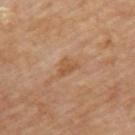Recorded during total-body skin imaging; not selected for excision or biopsy. Automated tile analysis of the lesion measured an area of roughly 3.5 mm², an outline eccentricity of about 0.8 (0 = round, 1 = elongated), and a symmetry-axis asymmetry near 0.45. And it measured a mean CIELAB color near L≈54 a*≈21 b*≈37 and roughly 8 lightness units darker than nearby skin. It also reported border irregularity of about 4.5 on a 0–10 scale, internal color variation of about 1 on a 0–10 scale, and a peripheral color-asymmetry measure near 0.5. The analysis additionally found a nevus-likeness score of about 0/100 and a detector confidence of about 100 out of 100 that the crop contains a lesion. About 3 mm across. The lesion is located on the upper back. A lesion tile, about 15 mm wide, cut from a 3D total-body photograph. Imaged with cross-polarized lighting.